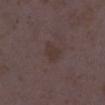Clinical impression:
This lesion was catalogued during total-body skin photography and was not selected for biopsy.
Acquisition and patient details:
A roughly 15 mm field-of-view crop from a total-body skin photograph. Approximately 2.5 mm at its widest. A female patient in their mid- to late 30s. From the right lower leg. Automated image analysis of the tile measured border irregularity of about 2 on a 0–10 scale and a peripheral color-asymmetry measure near 0.5.